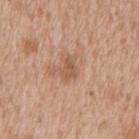Part of a total-body skin-imaging series; this lesion was reviewed on a skin check and was not flagged for biopsy.
A lesion tile, about 15 mm wide, cut from a 3D total-body photograph.
The subject is a male roughly 65 years of age.
Located on the mid back.